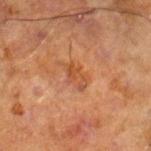biopsy_status: not biopsied; imaged during a skin examination